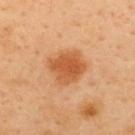Impression:
Part of a total-body skin-imaging series; this lesion was reviewed on a skin check and was not flagged for biopsy.